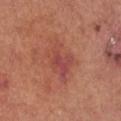Located on the front of the torso. An algorithmic analysis of the crop reported a mean CIELAB color near L≈48 a*≈29 b*≈28, about 7 CIELAB-L* units darker than the surrounding skin, and a normalized border contrast of about 6. A female patient, in their mid-60s. A lesion tile, about 15 mm wide, cut from a 3D total-body photograph. The lesion's longest dimension is about 5.5 mm. This is a white-light tile.